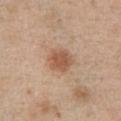Findings:
* follow-up · imaged on a skin check; not biopsied
* subject · female, aged 38 to 42
* image · ~15 mm tile from a whole-body skin photo
* site · the chest
* lesion size · about 3.5 mm